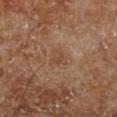Clinical impression: No biopsy was performed on this lesion — it was imaged during a full skin examination and was not determined to be concerning. Context: Approximately 2.5 mm at its widest. The total-body-photography lesion software estimated an average lesion color of about L≈44 a*≈19 b*≈30 (CIELAB), roughly 6 lightness units darker than nearby skin, and a lesion-to-skin contrast of about 5 (normalized; higher = more distinct). And it measured lesion-presence confidence of about 100/100. The lesion is on the leg. A male patient about 70 years old. A lesion tile, about 15 mm wide, cut from a 3D total-body photograph. The tile uses cross-polarized illumination.